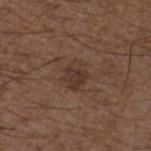No biopsy was performed on this lesion — it was imaged during a full skin examination and was not determined to be concerning.
On the chest.
A lesion tile, about 15 mm wide, cut from a 3D total-body photograph.
Automated image analysis of the tile measured a mean CIELAB color near L≈34 a*≈17 b*≈23, a lesion–skin lightness drop of about 7, and a lesion-to-skin contrast of about 6.5 (normalized; higher = more distinct). It also reported border irregularity of about 2.5 on a 0–10 scale and radial color variation of about 1.
A male subject, about 50 years old.